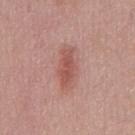Captured during whole-body skin photography for melanoma surveillance; the lesion was not biopsied. The lesion is located on the front of the torso. Longest diameter approximately 4.5 mm. Automated tile analysis of the lesion measured an average lesion color of about L≈53 a*≈26 b*≈25 (CIELAB), roughly 10 lightness units darker than nearby skin, and a lesion-to-skin contrast of about 7 (normalized; higher = more distinct). It also reported border irregularity of about 3 on a 0–10 scale, a within-lesion color-variation index near 2.5/10, and peripheral color asymmetry of about 1. Captured under white-light illumination. A male subject, approximately 45 years of age. A roughly 15 mm field-of-view crop from a total-body skin photograph.